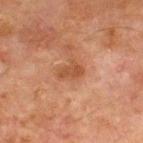Impression: This lesion was catalogued during total-body skin photography and was not selected for biopsy. Acquisition and patient details: Automated tile analysis of the lesion measured a lesion area of about 4 mm² and an eccentricity of roughly 0.85. The software also gave a mean CIELAB color near L≈40 a*≈21 b*≈30, about 7 CIELAB-L* units darker than the surrounding skin, and a normalized border contrast of about 7. The analysis additionally found an automated nevus-likeness rating near 0 out of 100 and a lesion-detection confidence of about 100/100. The subject is a male aged 63 to 67. Captured under cross-polarized illumination. About 3 mm across. The lesion is located on the chest. A 15 mm close-up tile from a total-body photography series done for melanoma screening.